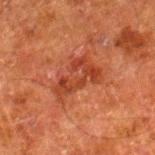Assessment:
This lesion was catalogued during total-body skin photography and was not selected for biopsy.
Acquisition and patient details:
This image is a 15 mm lesion crop taken from a total-body photograph. Approximately 5 mm at its widest. On the right lower leg. A male patient in their 80s.